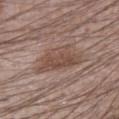Q: Is there a histopathology result?
A: catalogued during a skin exam; not biopsied
Q: What is the anatomic site?
A: the right forearm
Q: What kind of image is this?
A: ~15 mm tile from a whole-body skin photo
Q: Patient demographics?
A: male, aged 23–27